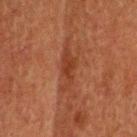Part of a total-body skin-imaging series; this lesion was reviewed on a skin check and was not flagged for biopsy. About 5 mm across. A roughly 15 mm field-of-view crop from a total-body skin photograph. The subject is a male aged 58–62. This is a cross-polarized tile. From the head or neck. The total-body-photography lesion software estimated a mean CIELAB color near L≈30 a*≈22 b*≈28, a lesion–skin lightness drop of about 7, and a normalized lesion–skin contrast near 6.5.